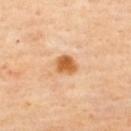Captured during whole-body skin photography for melanoma surveillance; the lesion was not biopsied. This image is a 15 mm lesion crop taken from a total-body photograph. The lesion is located on the upper back. A female subject, aged 58–62. Imaged with cross-polarized lighting. The lesion-visualizer software estimated an area of roughly 5.5 mm², an outline eccentricity of about 0.55 (0 = round, 1 = elongated), and two-axis asymmetry of about 0.2. The software also gave an average lesion color of about L≈63 a*≈25 b*≈44 (CIELAB). The software also gave a border-irregularity index near 2/10. The analysis additionally found lesion-presence confidence of about 100/100.